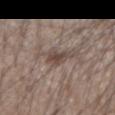Case summary:
– biopsy status — catalogued during a skin exam; not biopsied
– diameter — ~3 mm (longest diameter)
– lighting — white-light
– site — the left forearm
– acquisition — ~15 mm tile from a whole-body skin photo
– patient — male, aged approximately 55